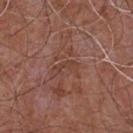Captured during whole-body skin photography for melanoma surveillance; the lesion was not biopsied.
A roughly 15 mm field-of-view crop from a total-body skin photograph.
The lesion is on the front of the torso.
A male subject roughly 55 years of age.